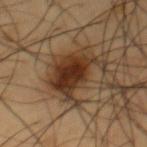<record>
  <biopsy_status>not biopsied; imaged during a skin examination</biopsy_status>
  <patient>
    <sex>male</sex>
    <age_approx>60</age_approx>
  </patient>
  <site>front of the torso</site>
  <lesion_size>
    <long_diameter_mm_approx>6.5</long_diameter_mm_approx>
  </lesion_size>
  <lighting>cross-polarized</lighting>
  <automated_metrics>
    <eccentricity>0.8</eccentricity>
    <shape_asymmetry>0.35</shape_asymmetry>
    <cielab_L>33</cielab_L>
    <cielab_a>18</cielab_a>
    <cielab_b>29</cielab_b>
    <vs_skin_darker_L>13.0</vs_skin_darker_L>
    <vs_skin_contrast_norm>12.0</vs_skin_contrast_norm>
    <border_irregularity_0_10>5.5</border_irregularity_0_10>
    <color_variation_0_10>6.0</color_variation_0_10>
    <peripheral_color_asymmetry>2.0</peripheral_color_asymmetry>
    <nevus_likeness_0_100>100</nevus_likeness_0_100>
    <lesion_detection_confidence_0_100>100</lesion_detection_confidence_0_100>
  </automated_metrics>
  <image>
    <source>total-body photography crop</source>
    <field_of_view_mm>15</field_of_view_mm>
  </image>
</record>